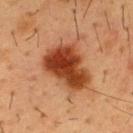The lesion was photographed on a routine skin check and not biopsied; there is no pathology result.
Located on the front of the torso.
The total-body-photography lesion software estimated a lesion color around L≈37 a*≈26 b*≈34 in CIELAB, roughly 15 lightness units darker than nearby skin, and a normalized lesion–skin contrast near 12.5.
A close-up tile cropped from a whole-body skin photograph, about 15 mm across.
Imaged with cross-polarized lighting.
The subject is a male aged approximately 55.
About 6 mm across.